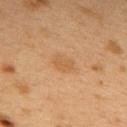subject: female, in their 40s | body site: the back | imaging modality: 15 mm crop, total-body photography | tile lighting: cross-polarized | automated lesion analysis: an area of roughly 5.5 mm² and a symmetry-axis asymmetry near 0.25; an average lesion color of about L≈47 a*≈17 b*≈33 (CIELAB) and a lesion–skin lightness drop of about 5; internal color variation of about 2 on a 0–10 scale and a peripheral color-asymmetry measure near 0.5; a classifier nevus-likeness of about 15/100 and a lesion-detection confidence of about 100/100 | size: ~3 mm (longest diameter).